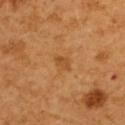Findings:
* biopsy status — total-body-photography surveillance lesion; no biopsy
* location — the upper back
* image source — 15 mm crop, total-body photography
* lighting — cross-polarized
* patient — female, aged 53–57